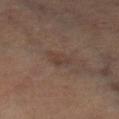Case summary:
– follow-up — catalogued during a skin exam; not biopsied
– image — ~15 mm tile from a whole-body skin photo
– TBP lesion metrics — a border-irregularity index near 3/10 and internal color variation of about 1 on a 0–10 scale; a detector confidence of about 100 out of 100 that the crop contains a lesion
– anatomic site — the right leg
– size — ~3 mm (longest diameter)
– illumination — cross-polarized illumination
– subject — female, aged 63–67